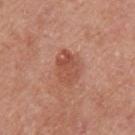A female patient, aged 38 to 42.
The tile uses white-light illumination.
The lesion is located on the left upper arm.
The recorded lesion diameter is about 4 mm.
Cropped from a total-body skin-imaging series; the visible field is about 15 mm.